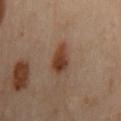Recorded during total-body skin imaging; not selected for excision or biopsy. On the mid back. This image is a 15 mm lesion crop taken from a total-body photograph. The patient is a male about 65 years old. This is a cross-polarized tile. About 4 mm across.